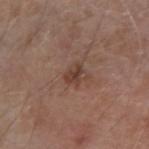workup = imaged on a skin check; not biopsied
lesion size = about 2.5 mm
image = ~15 mm crop, total-body skin-cancer survey
subject = male, aged 78 to 82
location = the left forearm
automated metrics = border irregularity of about 3.5 on a 0–10 scale and a peripheral color-asymmetry measure near 0.5; a classifier nevus-likeness of about 25/100
tile lighting = white-light illumination